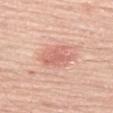Clinical impression: Recorded during total-body skin imaging; not selected for excision or biopsy.